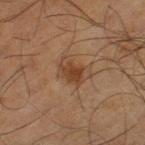{"biopsy_status": "not biopsied; imaged during a skin examination", "site": "leg", "automated_metrics": {"area_mm2_approx": 6.0, "eccentricity": 0.65, "shape_asymmetry": 0.25, "vs_skin_darker_L": 8.0, "vs_skin_contrast_norm": 7.5, "border_irregularity_0_10": 2.5, "color_variation_0_10": 2.5, "peripheral_color_asymmetry": 1.0, "nevus_likeness_0_100": 50, "lesion_detection_confidence_0_100": 100}, "lesion_size": {"long_diameter_mm_approx": 3.0}, "patient": {"sex": "male", "age_approx": 65}, "lighting": "cross-polarized", "image": {"source": "total-body photography crop", "field_of_view_mm": 15}}